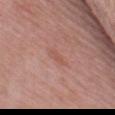Impression: This lesion was catalogued during total-body skin photography and was not selected for biopsy. Context: From the mid back. The total-body-photography lesion software estimated a border-irregularity rating of about 3/10 and a within-lesion color-variation index near 0/10. And it measured a classifier nevus-likeness of about 0/100 and a detector confidence of about 100 out of 100 that the crop contains a lesion. A 15 mm crop from a total-body photograph taken for skin-cancer surveillance. This is a white-light tile. The patient is a female aged 58–62.